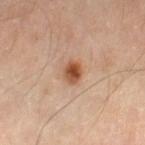Recorded during total-body skin imaging; not selected for excision or biopsy.
Approximately 2.5 mm at its widest.
A male subject, aged approximately 55.
Automated image analysis of the tile measured a lesion area of about 4.5 mm², a shape eccentricity near 0.6, and two-axis asymmetry of about 0.3. The software also gave a lesion color around L≈52 a*≈24 b*≈34 in CIELAB, about 14 CIELAB-L* units darker than the surrounding skin, and a lesion-to-skin contrast of about 10.5 (normalized; higher = more distinct).
Imaged with cross-polarized lighting.
Located on the right lower leg.
A lesion tile, about 15 mm wide, cut from a 3D total-body photograph.